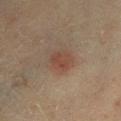Case summary:
– biopsy status · total-body-photography surveillance lesion; no biopsy
– subject · female, roughly 55 years of age
– lesion size · about 3.5 mm
– acquisition · 15 mm crop, total-body photography
– illumination · cross-polarized illumination
– location · the right lower leg